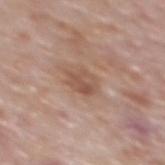Imaged during a routine full-body skin examination; the lesion was not biopsied and no histopathology is available. Approximately 3 mm at its widest. A male subject about 75 years old. A 15 mm close-up tile from a total-body photography series done for melanoma screening. This is a white-light tile. Located on the mid back. Automated tile analysis of the lesion measured an eccentricity of roughly 0.55 and two-axis asymmetry of about 0.2. The analysis additionally found a lesion color around L≈52 a*≈19 b*≈28 in CIELAB and a lesion-to-skin contrast of about 6.5 (normalized; higher = more distinct). The analysis additionally found a border-irregularity rating of about 2.5/10, a color-variation rating of about 3.5/10, and radial color variation of about 1.5. And it measured a classifier nevus-likeness of about 0/100 and a lesion-detection confidence of about 100/100.